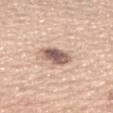biopsy status = catalogued during a skin exam; not biopsied | acquisition = ~15 mm tile from a whole-body skin photo | location = the right forearm | lesion size = about 3.5 mm | subject = female, in their mid-60s | automated metrics = a mean CIELAB color near L≈58 a*≈17 b*≈23, about 17 CIELAB-L* units darker than the surrounding skin, and a normalized lesion–skin contrast near 11; a border-irregularity index near 2/10, internal color variation of about 5.5 on a 0–10 scale, and a peripheral color-asymmetry measure near 1.5; a lesion-detection confidence of about 100/100 | tile lighting = white-light.